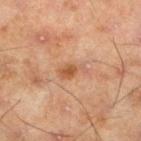Q: Was this lesion biopsied?
A: no biopsy performed (imaged during a skin exam)
Q: What is the lesion's diameter?
A: about 3 mm
Q: What is the imaging modality?
A: ~15 mm tile from a whole-body skin photo
Q: What did automated image analysis measure?
A: a footprint of about 4 mm², an outline eccentricity of about 0.85 (0 = round, 1 = elongated), and a symmetry-axis asymmetry near 0.4; border irregularity of about 4.5 on a 0–10 scale, internal color variation of about 2.5 on a 0–10 scale, and radial color variation of about 0.5
Q: Where on the body is the lesion?
A: the left lower leg
Q: What are the patient's age and sex?
A: male, in their mid-40s